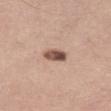acquisition = ~15 mm crop, total-body skin-cancer survey | illumination = white-light illumination | location = the left thigh | patient = female, roughly 45 years of age | lesion diameter = ≈3 mm.